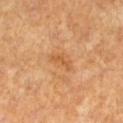Background: The subject is a female approximately 65 years of age. The lesion-visualizer software estimated border irregularity of about 6 on a 0–10 scale, a within-lesion color-variation index near 0/10, and a peripheral color-asymmetry measure near 0. The software also gave a nevus-likeness score of about 0/100 and a detector confidence of about 100 out of 100 that the crop contains a lesion. Approximately 3 mm at its widest. This image is a 15 mm lesion crop taken from a total-body photograph. The tile uses cross-polarized illumination. The lesion is on the left lower leg.